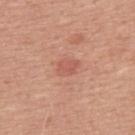Clinical impression: Part of a total-body skin-imaging series; this lesion was reviewed on a skin check and was not flagged for biopsy. Acquisition and patient details: A 15 mm close-up tile from a total-body photography series done for melanoma screening. Automated image analysis of the tile measured an area of roughly 4 mm², an eccentricity of roughly 0.75, and a shape-asymmetry score of about 0.2 (0 = symmetric). The analysis additionally found border irregularity of about 2 on a 0–10 scale, internal color variation of about 1.5 on a 0–10 scale, and radial color variation of about 0.5. It also reported lesion-presence confidence of about 100/100. The lesion's longest dimension is about 2.5 mm. The lesion is on the upper back. A male patient, approximately 50 years of age.